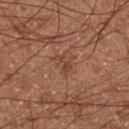Imaged during a routine full-body skin examination; the lesion was not biopsied and no histopathology is available. About 2.5 mm across. The lesion-visualizer software estimated an outline eccentricity of about 0.9 (0 = round, 1 = elongated) and a shape-asymmetry score of about 0.5 (0 = symmetric). The software also gave a lesion color around L≈38 a*≈20 b*≈27 in CIELAB and roughly 6 lightness units darker than nearby skin. The lesion is on the left lower leg. A 15 mm close-up extracted from a 3D total-body photography capture. This is a cross-polarized tile. A male patient in their 60s.